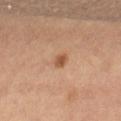Impression: The lesion was photographed on a routine skin check and not biopsied; there is no pathology result. Clinical summary: Captured under cross-polarized illumination. Cropped from a total-body skin-imaging series; the visible field is about 15 mm. Automated image analysis of the tile measured an area of roughly 2.5 mm² and a symmetry-axis asymmetry near 0.25. The lesion is on the right thigh. The recorded lesion diameter is about 2 mm. A female patient aged around 65.